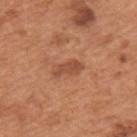  lighting: white-light
  site: back
  patient:
    sex: male
    age_approx: 65
  lesion_size:
    long_diameter_mm_approx: 4.0
  image:
    source: total-body photography crop
    field_of_view_mm: 15